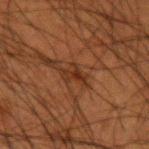follow-up: catalogued during a skin exam; not biopsied | anatomic site: the left upper arm | automated lesion analysis: a footprint of about 3.5 mm² and a shape-asymmetry score of about 0.6 (0 = symmetric) | patient: male, aged 48–52 | imaging modality: ~15 mm crop, total-body skin-cancer survey | lesion diameter: about 3 mm | illumination: cross-polarized illumination.